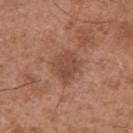Captured during whole-body skin photography for melanoma surveillance; the lesion was not biopsied.
The tile uses white-light illumination.
A region of skin cropped from a whole-body photographic capture, roughly 15 mm wide.
An algorithmic analysis of the crop reported a mean CIELAB color near L≈47 a*≈22 b*≈29, a lesion–skin lightness drop of about 8, and a normalized lesion–skin contrast near 6. It also reported a border-irregularity rating of about 2.5/10 and internal color variation of about 2.5 on a 0–10 scale.
From the right upper arm.
A male subject aged 48 to 52.
Longest diameter approximately 3.5 mm.